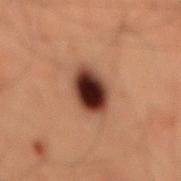Notes:
• biopsy status: total-body-photography surveillance lesion; no biopsy
• image source: total-body-photography crop, ~15 mm field of view
• automated lesion analysis: an average lesion color of about L≈26 a*≈18 b*≈21 (CIELAB) and a lesion-to-skin contrast of about 17 (normalized; higher = more distinct); a border-irregularity index near 1.5/10 and peripheral color asymmetry of about 2; an automated nevus-likeness rating near 100 out of 100 and lesion-presence confidence of about 100/100
• size: ~4.5 mm (longest diameter)
• body site: the mid back
• subject: male, in their 60s
• lighting: cross-polarized